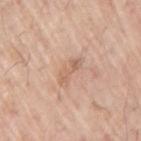Impression:
No biopsy was performed on this lesion — it was imaged during a full skin examination and was not determined to be concerning.
Acquisition and patient details:
On the right upper arm. A close-up tile cropped from a whole-body skin photograph, about 15 mm across. The recorded lesion diameter is about 3.5 mm. The subject is a male in their 70s.